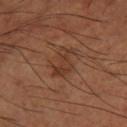biopsy status: total-body-photography surveillance lesion; no biopsy
tile lighting: cross-polarized illumination
patient: male, in their mid- to late 60s
lesion size: about 4 mm
site: the right thigh
acquisition: total-body-photography crop, ~15 mm field of view
automated metrics: a footprint of about 9 mm², an outline eccentricity of about 0.65 (0 = round, 1 = elongated), and two-axis asymmetry of about 0.35; a border-irregularity rating of about 4/10 and a within-lesion color-variation index near 3/10; an automated nevus-likeness rating near 10 out of 100